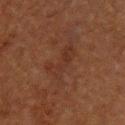Assessment: Recorded during total-body skin imaging; not selected for excision or biopsy. Acquisition and patient details: A male subject aged approximately 50. The tile uses cross-polarized illumination. The lesion is located on the upper back. The recorded lesion diameter is about 4.5 mm. The lesion-visualizer software estimated a footprint of about 6.5 mm², an outline eccentricity of about 0.9 (0 = round, 1 = elongated), and two-axis asymmetry of about 0.45. It also reported an average lesion color of about L≈25 a*≈18 b*≈23 (CIELAB), roughly 4 lightness units darker than nearby skin, and a normalized lesion–skin contrast near 5. The software also gave a classifier nevus-likeness of about 0/100 and lesion-presence confidence of about 100/100. Cropped from a total-body skin-imaging series; the visible field is about 15 mm.